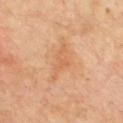Case summary:
– follow-up: total-body-photography surveillance lesion; no biopsy
– patient: male, approximately 70 years of age
– image-analysis metrics: a lesion color around L≈61 a*≈23 b*≈38 in CIELAB, about 7 CIELAB-L* units darker than the surrounding skin, and a normalized lesion–skin contrast near 5; border irregularity of about 5.5 on a 0–10 scale, internal color variation of about 2 on a 0–10 scale, and a peripheral color-asymmetry measure near 0.5
– illumination: cross-polarized
– image: ~15 mm crop, total-body skin-cancer survey
– diameter: ≈5 mm
– anatomic site: the chest